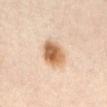Case summary:
• workup — imaged on a skin check; not biopsied
• lesion size — about 4 mm
• acquisition — total-body-photography crop, ~15 mm field of view
• patient — female, aged around 40
• body site — the front of the torso
• lighting — cross-polarized illumination
• TBP lesion metrics — an area of roughly 11 mm² and a shape eccentricity near 0.65; an average lesion color of about L≈65 a*≈19 b*≈36 (CIELAB) and a normalized border contrast of about 10; a border-irregularity rating of about 1.5/10 and peripheral color asymmetry of about 2